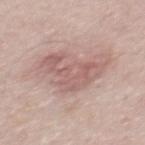This lesion was catalogued during total-body skin photography and was not selected for biopsy.
Measured at roughly 6 mm in maximum diameter.
A male subject, in their mid- to late 40s.
A roughly 15 mm field-of-view crop from a total-body skin photograph.
Captured under white-light illumination.
The lesion is located on the mid back.